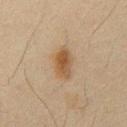{"biopsy_status": "not biopsied; imaged during a skin examination", "lesion_size": {"long_diameter_mm_approx": 4.5}, "lighting": "cross-polarized", "patient": {"sex": "male", "age_approx": 65}, "site": "front of the torso", "image": {"source": "total-body photography crop", "field_of_view_mm": 15}}